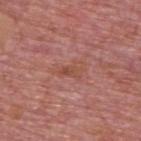Part of a total-body skin-imaging series; this lesion was reviewed on a skin check and was not flagged for biopsy.
Cropped from a whole-body photographic skin survey; the tile spans about 15 mm.
Measured at roughly 3.5 mm in maximum diameter.
This is a white-light tile.
On the upper back.
The total-body-photography lesion software estimated an outline eccentricity of about 0.85 (0 = round, 1 = elongated) and two-axis asymmetry of about 0.45. And it measured border irregularity of about 5.5 on a 0–10 scale, internal color variation of about 2.5 on a 0–10 scale, and radial color variation of about 0.5. And it measured an automated nevus-likeness rating near 0 out of 100 and a lesion-detection confidence of about 95/100.
The subject is a male aged 73 to 77.